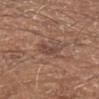Recorded during total-body skin imaging; not selected for excision or biopsy. The lesion is located on the left forearm. Automated image analysis of the tile measured an average lesion color of about L≈45 a*≈19 b*≈24 (CIELAB), about 7 CIELAB-L* units darker than the surrounding skin, and a normalized lesion–skin contrast near 6. A male patient, in their mid- to late 60s. The lesion's longest dimension is about 3 mm. A region of skin cropped from a whole-body photographic capture, roughly 15 mm wide.